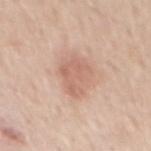<lesion>
  <biopsy_status>not biopsied; imaged during a skin examination</biopsy_status>
  <automated_metrics>
    <area_mm2_approx>11.0</area_mm2_approx>
    <eccentricity>0.75</eccentricity>
    <shape_asymmetry>0.25</shape_asymmetry>
  </automated_metrics>
  <patient>
    <sex>male</sex>
    <age_approx>60</age_approx>
  </patient>
  <site>mid back</site>
  <lighting>white-light</lighting>
  <lesion_size>
    <long_diameter_mm_approx>4.5</long_diameter_mm_approx>
  </lesion_size>
  <image>
    <source>total-body photography crop</source>
    <field_of_view_mm>15</field_of_view_mm>
  </image>
</lesion>